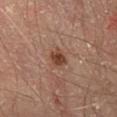Imaged during a routine full-body skin examination; the lesion was not biopsied and no histopathology is available. The subject is a male approximately 65 years of age. The lesion is located on the right thigh. A region of skin cropped from a whole-body photographic capture, roughly 15 mm wide.